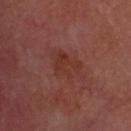Impression:
The lesion was tiled from a total-body skin photograph and was not biopsied.
Image and clinical context:
Automated tile analysis of the lesion measured a footprint of about 11 mm², a shape eccentricity near 0.8, and a symmetry-axis asymmetry near 0.35. The software also gave an average lesion color of about L≈30 a*≈22 b*≈23 (CIELAB), about 4 CIELAB-L* units darker than the surrounding skin, and a lesion-to-skin contrast of about 5 (normalized; higher = more distinct). The analysis additionally found a classifier nevus-likeness of about 0/100 and lesion-presence confidence of about 100/100. A male subject, aged 58 to 62. A 15 mm close-up tile from a total-body photography series done for melanoma screening. Approximately 5.5 mm at its widest. From the head or neck.